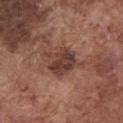notes — imaged on a skin check; not biopsied
size — about 4 mm
acquisition — 15 mm crop, total-body photography
illumination — white-light illumination
TBP lesion metrics — a lesion area of about 10 mm², an outline eccentricity of about 0.5 (0 = round, 1 = elongated), and a symmetry-axis asymmetry near 0.3; a border-irregularity rating of about 4/10, a within-lesion color-variation index near 3.5/10, and radial color variation of about 1.5; an automated nevus-likeness rating near 25 out of 100 and a detector confidence of about 100 out of 100 that the crop contains a lesion
anatomic site — the chest
patient — male, aged 73–77